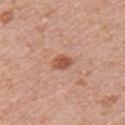* notes — total-body-photography surveillance lesion; no biopsy
* subject — female, aged approximately 50
* location — the chest
* image — 15 mm crop, total-body photography